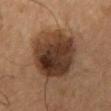Findings:
• biopsy status · no biopsy performed (imaged during a skin exam)
• TBP lesion metrics · border irregularity of about 2 on a 0–10 scale, a color-variation rating of about 7.5/10, and peripheral color asymmetry of about 2.5; an automated nevus-likeness rating near 75 out of 100
• subject · male, aged 53 to 57
• imaging modality · ~15 mm crop, total-body skin-cancer survey
• lesion diameter · about 8 mm
• body site · the chest
• tile lighting · cross-polarized